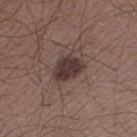Imaged during a routine full-body skin examination; the lesion was not biopsied and no histopathology is available.
From the left thigh.
The total-body-photography lesion software estimated a lesion area of about 8 mm², an outline eccentricity of about 0.65 (0 = round, 1 = elongated), and a symmetry-axis asymmetry near 0.25. And it measured an average lesion color of about L≈35 a*≈15 b*≈18 (CIELAB), a lesion–skin lightness drop of about 12, and a normalized border contrast of about 10.5. The analysis additionally found a border-irregularity rating of about 2.5/10, a color-variation rating of about 2.5/10, and a peripheral color-asymmetry measure near 1. The analysis additionally found an automated nevus-likeness rating near 50 out of 100 and lesion-presence confidence of about 100/100.
Captured under white-light illumination.
A male subject aged around 35.
Approximately 3.5 mm at its widest.
A 15 mm close-up tile from a total-body photography series done for melanoma screening.